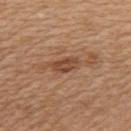biopsy status = imaged on a skin check; not biopsied | lesion size = ~3.5 mm (longest diameter) | illumination = white-light | site = the left upper arm | image source = ~15 mm tile from a whole-body skin photo | patient = female, aged around 55.